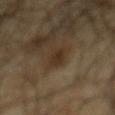Notes:
– workup — no biopsy performed (imaged during a skin exam)
– patient — male, aged around 65
– site — the mid back
– acquisition — ~15 mm tile from a whole-body skin photo
– tile lighting — cross-polarized illumination